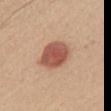Clinical impression:
Imaged during a routine full-body skin examination; the lesion was not biopsied and no histopathology is available.
Background:
The lesion is located on the head or neck. Longest diameter approximately 4 mm. This image is a 15 mm lesion crop taken from a total-body photograph. The total-body-photography lesion software estimated a border-irregularity index near 1/10, a within-lesion color-variation index near 4/10, and radial color variation of about 1. The analysis additionally found an automated nevus-likeness rating near 100 out of 100 and a detector confidence of about 100 out of 100 that the crop contains a lesion. A female subject roughly 45 years of age.